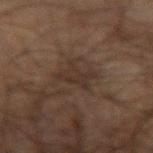workup: catalogued during a skin exam; not biopsied | location: the left forearm | acquisition: total-body-photography crop, ~15 mm field of view | size: about 4 mm | automated lesion analysis: an area of roughly 7 mm² and a shape-asymmetry score of about 0.4 (0 = symmetric) | patient: male, aged 68 to 72 | tile lighting: cross-polarized.